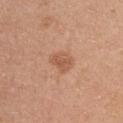This lesion was catalogued during total-body skin photography and was not selected for biopsy. Cropped from a whole-body photographic skin survey; the tile spans about 15 mm. Located on the chest. A female patient in their mid- to late 40s.